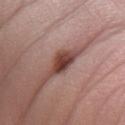No biopsy was performed on this lesion — it was imaged during a full skin examination and was not determined to be concerning. The total-body-photography lesion software estimated about 16 CIELAB-L* units darker than the surrounding skin and a normalized lesion–skin contrast near 12.5. And it measured a color-variation rating of about 3.5/10 and peripheral color asymmetry of about 1. This is a white-light tile. From the right forearm. A female subject, about 50 years old. This image is a 15 mm lesion crop taken from a total-body photograph.